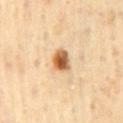notes: no biopsy performed (imaged during a skin exam); body site: the mid back; subject: male, about 65 years old; diameter: about 3 mm; image source: ~15 mm crop, total-body skin-cancer survey; tile lighting: cross-polarized illumination.